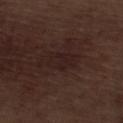The subject is a male aged approximately 70. From the left lower leg. Automated tile analysis of the lesion measured a footprint of about 5.5 mm², an eccentricity of roughly 0.8, and two-axis asymmetry of about 0.25. It also reported a mean CIELAB color near L≈19 a*≈16 b*≈16 and roughly 4 lightness units darker than nearby skin. It also reported internal color variation of about 2 on a 0–10 scale and radial color variation of about 0.5. This is a white-light tile. A 15 mm close-up tile from a total-body photography series done for melanoma screening. The recorded lesion diameter is about 3.5 mm.